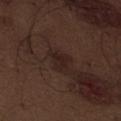No biopsy was performed on this lesion — it was imaged during a full skin examination and was not determined to be concerning. A male patient in their 70s. The lesion is on the abdomen. Automated image analysis of the tile measured an area of roughly 4 mm² and an eccentricity of roughly 0.75. The software also gave a lesion color around L≈21 a*≈16 b*≈17 in CIELAB and about 6 CIELAB-L* units darker than the surrounding skin. The analysis additionally found border irregularity of about 4 on a 0–10 scale. Cropped from a whole-body photographic skin survey; the tile spans about 15 mm. Captured under white-light illumination.